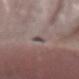Recorded during total-body skin imaging; not selected for excision or biopsy.
The lesion is located on the right forearm.
A lesion tile, about 15 mm wide, cut from a 3D total-body photograph.
Imaged with white-light lighting.
Longest diameter approximately 2.5 mm.
The subject is a male roughly 65 years of age.